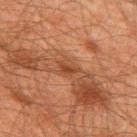workup = no biopsy performed (imaged during a skin exam) | lesion size = about 2.5 mm | imaging modality = total-body-photography crop, ~15 mm field of view | site = the upper back | patient = male, aged around 60 | automated lesion analysis = a lesion color around L≈34 a*≈21 b*≈28 in CIELAB, roughly 8 lightness units darker than nearby skin, and a normalized lesion–skin contrast near 7; border irregularity of about 4 on a 0–10 scale, a within-lesion color-variation index near 0.5/10, and radial color variation of about 0; an automated nevus-likeness rating near 0 out of 100 and a detector confidence of about 75 out of 100 that the crop contains a lesion.